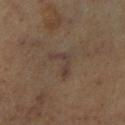biopsy status = imaged on a skin check; not biopsied.